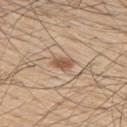Imaged with white-light lighting. On the upper back. The lesion's longest dimension is about 3 mm. An algorithmic analysis of the crop reported a lesion area of about 4 mm² and an eccentricity of roughly 0.85. The analysis additionally found an average lesion color of about L≈55 a*≈17 b*≈32 (CIELAB) and a lesion-to-skin contrast of about 8 (normalized; higher = more distinct). It also reported a border-irregularity rating of about 2.5/10, a color-variation rating of about 1.5/10, and peripheral color asymmetry of about 0.5. The subject is a male in their mid- to late 50s. A lesion tile, about 15 mm wide, cut from a 3D total-body photograph.